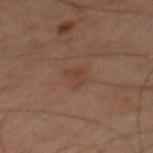Findings:
- notes · no biopsy performed (imaged during a skin exam)
- subject · male, about 60 years old
- size · ≈3 mm
- image · 15 mm crop, total-body photography
- body site · the mid back
- image-analysis metrics · a lesion area of about 4.5 mm² and a shape-asymmetry score of about 0.4 (0 = symmetric); roughly 4 lightness units darker than nearby skin and a normalized lesion–skin contrast near 4.5; a border-irregularity index near 4/10 and internal color variation of about 2 on a 0–10 scale